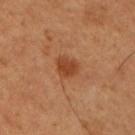Findings:
* notes: catalogued during a skin exam; not biopsied
* lighting: cross-polarized
* subject: male, roughly 50 years of age
* imaging modality: total-body-photography crop, ~15 mm field of view
* body site: the arm
* size: ~3 mm (longest diameter)